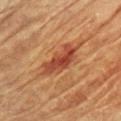Findings:
- workup — total-body-photography surveillance lesion; no biopsy
- lesion diameter — ≈5.5 mm
- location — the chest
- acquisition — ~15 mm tile from a whole-body skin photo
- subject — female, aged approximately 80